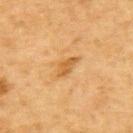This lesion was catalogued during total-body skin photography and was not selected for biopsy.
A region of skin cropped from a whole-body photographic capture, roughly 15 mm wide.
This is a cross-polarized tile.
A male subject, aged 83 or over.
The lesion is located on the upper back.
The lesion's longest dimension is about 3 mm.